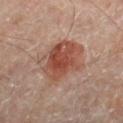biopsy_status: not biopsied; imaged during a skin examination
lesion_size:
  long_diameter_mm_approx: 5.5
patient:
  sex: male
  age_approx: 65
site: right lower leg
image:
  source: total-body photography crop
  field_of_view_mm: 15